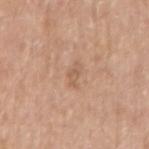This lesion was catalogued during total-body skin photography and was not selected for biopsy.
The lesion's longest dimension is about 2.5 mm.
A roughly 15 mm field-of-view crop from a total-body skin photograph.
On the arm.
This is a white-light tile.
The patient is a male aged around 70.